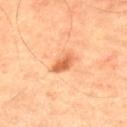workup: no biopsy performed (imaged during a skin exam) | patient: male, aged around 65 | acquisition: ~15 mm crop, total-body skin-cancer survey | anatomic site: the back.